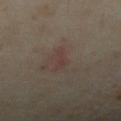Assessment:
Recorded during total-body skin imaging; not selected for excision or biopsy.
Context:
A female patient, aged around 35. The lesion's longest dimension is about 2.5 mm. The lesion is located on the leg. A close-up tile cropped from a whole-body skin photograph, about 15 mm across.